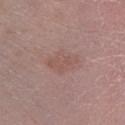Impression: The lesion was photographed on a routine skin check and not biopsied; there is no pathology result. Clinical summary: A female subject aged around 60. A lesion tile, about 15 mm wide, cut from a 3D total-body photograph. Automated tile analysis of the lesion measured an area of roughly 7.5 mm² and an eccentricity of roughly 0.75. The analysis additionally found an average lesion color of about L≈53 a*≈20 b*≈22 (CIELAB) and a lesion-to-skin contrast of about 4.5 (normalized; higher = more distinct). It also reported internal color variation of about 2 on a 0–10 scale and peripheral color asymmetry of about 1. The analysis additionally found a classifier nevus-likeness of about 0/100 and lesion-presence confidence of about 100/100. From the right lower leg. The recorded lesion diameter is about 4 mm.